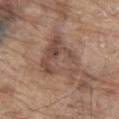Imaged with white-light lighting. The lesion is on the abdomen. The subject is a male aged approximately 80. The lesion's longest dimension is about 6.5 mm. A 15 mm close-up extracted from a 3D total-body photography capture.